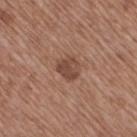notes: no biopsy performed (imaged during a skin exam) | subject: female, in their mid- to late 70s | image-analysis metrics: a footprint of about 5 mm², a shape eccentricity near 0.45, and a shape-asymmetry score of about 0.25 (0 = symmetric); a border-irregularity index near 2/10 and radial color variation of about 1; a classifier nevus-likeness of about 10/100 | image: total-body-photography crop, ~15 mm field of view | lighting: white-light illumination | site: the leg.